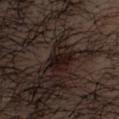This lesion was catalogued during total-body skin photography and was not selected for biopsy. Located on the head or neck. Captured under cross-polarized illumination. A close-up tile cropped from a whole-body skin photograph, about 15 mm across. A male subject about 40 years old. Longest diameter approximately 3 mm.